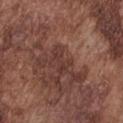workup = no biopsy performed (imaged during a skin exam).